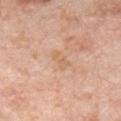Assessment:
Recorded during total-body skin imaging; not selected for excision or biopsy.
Context:
The lesion is on the front of the torso. A male patient roughly 60 years of age. A lesion tile, about 15 mm wide, cut from a 3D total-body photograph. Measured at roughly 2.5 mm in maximum diameter. This is a white-light tile.